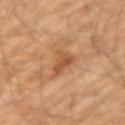Notes:
- workup · total-body-photography surveillance lesion; no biopsy
- TBP lesion metrics · an area of roughly 5 mm² and an outline eccentricity of about 0.85 (0 = round, 1 = elongated)
- patient · male, in their mid-60s
- lighting · cross-polarized
- site · the left upper arm
- diameter · ≈4 mm
- imaging modality · 15 mm crop, total-body photography